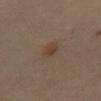Q: Was this lesion biopsied?
A: imaged on a skin check; not biopsied
Q: Where on the body is the lesion?
A: the abdomen
Q: What is the imaging modality?
A: ~15 mm tile from a whole-body skin photo
Q: Illumination type?
A: cross-polarized
Q: Patient demographics?
A: female, aged 63–67